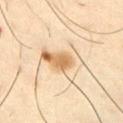follow-up=catalogued during a skin exam; not biopsied | anatomic site=the left upper arm | patient=male, in their 40s | size=≈5.5 mm | image=~15 mm crop, total-body skin-cancer survey | automated metrics=a shape eccentricity near 0.8; peripheral color asymmetry of about 4.